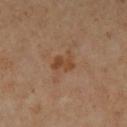Image and clinical context: Cropped from a whole-body photographic skin survey; the tile spans about 15 mm. A female subject, approximately 50 years of age. From the left lower leg. Longest diameter approximately 3 mm.